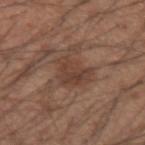This lesion was catalogued during total-body skin photography and was not selected for biopsy.
The total-body-photography lesion software estimated an area of roughly 9.5 mm² and an outline eccentricity of about 0.55 (0 = round, 1 = elongated).
A roughly 15 mm field-of-view crop from a total-body skin photograph.
The patient is a male approximately 35 years of age.
About 4 mm across.
Located on the left forearm.